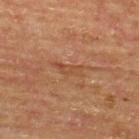Clinical impression: Recorded during total-body skin imaging; not selected for excision or biopsy. Image and clinical context: The subject is a male aged approximately 65. The lesion is on the back. A 15 mm close-up extracted from a 3D total-body photography capture. Imaged with cross-polarized lighting. The recorded lesion diameter is about 4 mm.